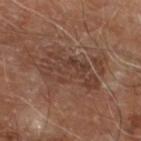Recorded during total-body skin imaging; not selected for excision or biopsy.